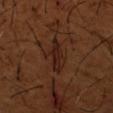Clinical impression:
The lesion was photographed on a routine skin check and not biopsied; there is no pathology result.
Image and clinical context:
A lesion tile, about 15 mm wide, cut from a 3D total-body photograph. A male subject, roughly 65 years of age. The lesion is on the arm. The tile uses cross-polarized illumination. The recorded lesion diameter is about 4.5 mm.